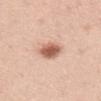Acquisition and patient details: The lesion is on the upper back. Captured under white-light illumination. The patient is a female about 40 years old. A 15 mm crop from a total-body photograph taken for skin-cancer surveillance. The recorded lesion diameter is about 3.5 mm.